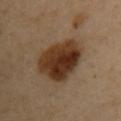Q: Was a biopsy performed?
A: total-body-photography surveillance lesion; no biopsy
Q: What is the imaging modality?
A: 15 mm crop, total-body photography
Q: What lighting was used for the tile?
A: cross-polarized illumination
Q: What is the anatomic site?
A: the left arm
Q: Who is the patient?
A: female, aged around 45
Q: Automated lesion metrics?
A: a lesion area of about 21 mm², a shape eccentricity near 0.75, and a shape-asymmetry score of about 0.2 (0 = symmetric)
Q: How large is the lesion?
A: about 6.5 mm